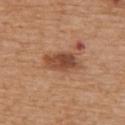Imaged during a routine full-body skin examination; the lesion was not biopsied and no histopathology is available.
Imaged with white-light lighting.
A male patient, roughly 70 years of age.
Approximately 4.5 mm at its widest.
Cropped from a whole-body photographic skin survey; the tile spans about 15 mm.
From the back.
Automated tile analysis of the lesion measured a lesion area of about 9.5 mm², an outline eccentricity of about 0.8 (0 = round, 1 = elongated), and a symmetry-axis asymmetry near 0.2. And it measured an average lesion color of about L≈48 a*≈23 b*≈32 (CIELAB), a lesion–skin lightness drop of about 12, and a lesion-to-skin contrast of about 8.5 (normalized; higher = more distinct). And it measured a color-variation rating of about 5/10 and radial color variation of about 1.5.